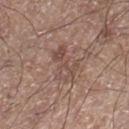Impression:
Recorded during total-body skin imaging; not selected for excision or biopsy.
Clinical summary:
A 15 mm close-up extracted from a 3D total-body photography capture. The lesion is on the right lower leg. The lesion's longest dimension is about 4 mm. A male subject aged around 55.